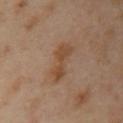The lesion was tiled from a total-body skin photograph and was not biopsied. Automated image analysis of the tile measured a lesion area of about 8 mm², an eccentricity of roughly 0.9, and a symmetry-axis asymmetry near 0.3. And it measured an average lesion color of about L≈48 a*≈18 b*≈32 (CIELAB) and a lesion–skin lightness drop of about 7. It also reported a border-irregularity rating of about 4.5/10 and a within-lesion color-variation index near 3/10. And it measured a classifier nevus-likeness of about 0/100 and a detector confidence of about 100 out of 100 that the crop contains a lesion. The lesion is on the chest. Imaged with cross-polarized lighting. Longest diameter approximately 5 mm. Cropped from a total-body skin-imaging series; the visible field is about 15 mm. A female patient, roughly 40 years of age.